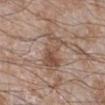{
  "biopsy_status": "not biopsied; imaged during a skin examination",
  "image": {
    "source": "total-body photography crop",
    "field_of_view_mm": 15
  },
  "lighting": "white-light",
  "site": "right lower leg",
  "patient": {
    "sex": "male",
    "age_approx": 60
  },
  "lesion_size": {
    "long_diameter_mm_approx": 5.5
  }
}